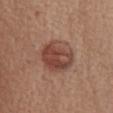biopsy_status: not biopsied; imaged during a skin examination
site: abdomen
patient:
  sex: female
  age_approx: 75
automated_metrics:
  eccentricity: 0.35
  shape_asymmetry: 0.1
  border_irregularity_0_10: 1.5
  color_variation_0_10: 6.0
  peripheral_color_asymmetry: 2.0
  lesion_detection_confidence_0_100: 100
image:
  source: total-body photography crop
  field_of_view_mm: 15
lesion_size:
  long_diameter_mm_approx: 4.5
lighting: white-light